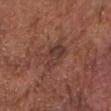Q: Was this lesion biopsied?
A: imaged on a skin check; not biopsied
Q: What are the patient's age and sex?
A: male, approximately 75 years of age
Q: What kind of image is this?
A: ~15 mm tile from a whole-body skin photo
Q: Lesion location?
A: the chest